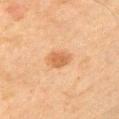A male subject approximately 65 years of age. The tile uses cross-polarized illumination. From the left upper arm. Approximately 2.5 mm at its widest. A close-up tile cropped from a whole-body skin photograph, about 15 mm across. Automated tile analysis of the lesion measured a lesion area of about 4.5 mm², a shape eccentricity near 0.45, and a shape-asymmetry score of about 0.2 (0 = symmetric). And it measured a mean CIELAB color near L≈50 a*≈20 b*≈34, about 9 CIELAB-L* units darker than the surrounding skin, and a normalized border contrast of about 7. The software also gave an automated nevus-likeness rating near 85 out of 100.